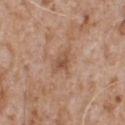workup = total-body-photography surveillance lesion; no biopsy | imaging modality = ~15 mm tile from a whole-body skin photo | lighting = white-light | body site = the chest | diameter = ~2.5 mm (longest diameter) | subject = male, aged 63–67.